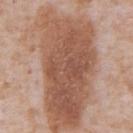biopsy status: imaged on a skin check; not biopsied
tile lighting: white-light
site: the front of the torso
acquisition: total-body-photography crop, ~15 mm field of view
subject: male, in their mid- to late 60s
image-analysis metrics: an outline eccentricity of about 0.85 (0 = round, 1 = elongated) and a symmetry-axis asymmetry near 0.3; an average lesion color of about L≈54 a*≈21 b*≈30 (CIELAB), roughly 13 lightness units darker than nearby skin, and a normalized lesion–skin contrast near 9
diameter: ~14 mm (longest diameter)